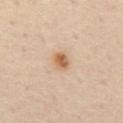Q: Is there a histopathology result?
A: catalogued during a skin exam; not biopsied
Q: What are the patient's age and sex?
A: male, aged 53 to 57
Q: What kind of image is this?
A: total-body-photography crop, ~15 mm field of view
Q: Lesion location?
A: the abdomen